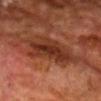| key | value |
|---|---|
| lighting | cross-polarized |
| subject | male, aged around 70 |
| site | the chest |
| imaging modality | ~15 mm tile from a whole-body skin photo |